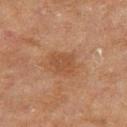The lesion was photographed on a routine skin check and not biopsied; there is no pathology result. A female subject, about 60 years old. On the left thigh. Imaged with cross-polarized lighting. Cropped from a total-body skin-imaging series; the visible field is about 15 mm. About 3 mm across.